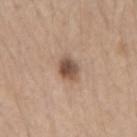{"biopsy_status": "not biopsied; imaged during a skin examination", "lesion_size": {"long_diameter_mm_approx": 2.5}, "image": {"source": "total-body photography crop", "field_of_view_mm": 15}, "patient": {"sex": "female", "age_approx": 45}, "automated_metrics": {"vs_skin_darker_L": 14.0, "vs_skin_contrast_norm": 9.5, "nevus_likeness_0_100": 75, "lesion_detection_confidence_0_100": 100}, "lighting": "white-light", "site": "left forearm"}